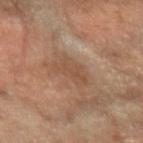A close-up tile cropped from a whole-body skin photograph, about 15 mm across.
The lesion is on the left forearm.
Imaged with cross-polarized lighting.
Longest diameter approximately 4 mm.
A male subject aged 58–62.
The lesion-visualizer software estimated a footprint of about 7 mm², an outline eccentricity of about 0.85 (0 = round, 1 = elongated), and a symmetry-axis asymmetry near 0.3. The analysis additionally found a mean CIELAB color near L≈46 a*≈18 b*≈29, about 6 CIELAB-L* units darker than the surrounding skin, and a lesion-to-skin contrast of about 5.5 (normalized; higher = more distinct). And it measured a border-irregularity index near 3.5/10, a color-variation rating of about 2/10, and radial color variation of about 0.5. The analysis additionally found a nevus-likeness score of about 0/100 and a lesion-detection confidence of about 100/100.